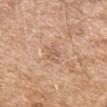Clinical impression:
Part of a total-body skin-imaging series; this lesion was reviewed on a skin check and was not flagged for biopsy.
Acquisition and patient details:
The lesion's longest dimension is about 2.5 mm. Automated tile analysis of the lesion measured an area of roughly 3 mm² and an outline eccentricity of about 0.65 (0 = round, 1 = elongated). The analysis additionally found roughly 7 lightness units darker than nearby skin and a normalized lesion–skin contrast near 5. And it measured an automated nevus-likeness rating near 0 out of 100 and lesion-presence confidence of about 100/100. A 15 mm close-up extracted from a 3D total-body photography capture. A male subject about 75 years old. The lesion is on the right upper arm.